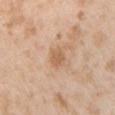Impression:
The lesion was tiled from a total-body skin photograph and was not biopsied.
Image and clinical context:
The tile uses white-light illumination. About 2.5 mm across. A roughly 15 mm field-of-view crop from a total-body skin photograph. A male patient, aged 23–27. The lesion is on the chest.